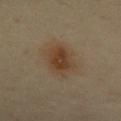The lesion was photographed on a routine skin check and not biopsied; there is no pathology result. Imaged with cross-polarized lighting. This image is a 15 mm lesion crop taken from a total-body photograph. About 5 mm across. The patient is a female about 45 years old. Automated tile analysis of the lesion measured a lesion area of about 13 mm², an outline eccentricity of about 0.7 (0 = round, 1 = elongated), and a shape-asymmetry score of about 0.15 (0 = symmetric). And it measured a mean CIELAB color near L≈41 a*≈15 b*≈30, about 8 CIELAB-L* units darker than the surrounding skin, and a normalized lesion–skin contrast near 8. The software also gave a border-irregularity index near 2/10, internal color variation of about 4 on a 0–10 scale, and a peripheral color-asymmetry measure near 1. It also reported an automated nevus-likeness rating near 95 out of 100 and lesion-presence confidence of about 100/100. Located on the chest.